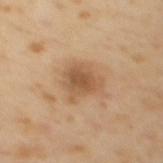Assessment: This lesion was catalogued during total-body skin photography and was not selected for biopsy.